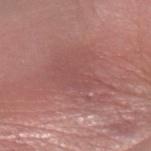workup = imaged on a skin check; not biopsied.